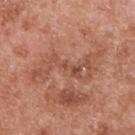No biopsy was performed on this lesion — it was imaged during a full skin examination and was not determined to be concerning. This is a white-light tile. The lesion-visualizer software estimated a classifier nevus-likeness of about 0/100 and a detector confidence of about 95 out of 100 that the crop contains a lesion. Cropped from a whole-body photographic skin survey; the tile spans about 15 mm. The lesion is located on the upper back. A male subject, aged 53–57. Approximately 8.5 mm at its widest.